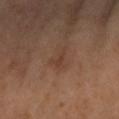The lesion was tiled from a total-body skin photograph and was not biopsied.
The patient is a male roughly 55 years of age.
The total-body-photography lesion software estimated a lesion–skin lightness drop of about 6 and a normalized border contrast of about 5.5. The analysis additionally found a border-irregularity index near 6/10, a color-variation rating of about 0/10, and a peripheral color-asymmetry measure near 0. The analysis additionally found an automated nevus-likeness rating near 0 out of 100 and a lesion-detection confidence of about 100/100.
This is a cross-polarized tile.
A 15 mm crop from a total-body photograph taken for skin-cancer surveillance.
On the right forearm.
Approximately 2.5 mm at its widest.